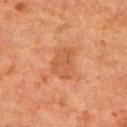Q: Was a biopsy performed?
A: catalogued during a skin exam; not biopsied
Q: What is the anatomic site?
A: the upper back
Q: What kind of image is this?
A: ~15 mm tile from a whole-body skin photo
Q: Who is the patient?
A: male, in their 80s